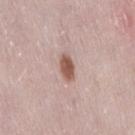Q: Illumination type?
A: white-light
Q: How was this image acquired?
A: ~15 mm tile from a whole-body skin photo
Q: Lesion location?
A: the right thigh
Q: Lesion size?
A: ≈3 mm
Q: Patient demographics?
A: female, roughly 30 years of age
Q: What did automated image analysis measure?
A: an area of roughly 5 mm², an outline eccentricity of about 0.8 (0 = round, 1 = elongated), and a symmetry-axis asymmetry near 0.2; a border-irregularity rating of about 1.5/10, internal color variation of about 3 on a 0–10 scale, and radial color variation of about 1